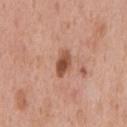| key | value |
|---|---|
| lighting | white-light illumination |
| image-analysis metrics | an area of roughly 4.5 mm², an outline eccentricity of about 0.9 (0 = round, 1 = elongated), and a symmetry-axis asymmetry near 0.3; a border-irregularity index near 3/10 |
| patient | male, roughly 55 years of age |
| location | the chest |
| imaging modality | ~15 mm crop, total-body skin-cancer survey |